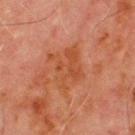biopsy_status: not biopsied; imaged during a skin examination
patient:
  sex: male
  age_approx: 70
site: chest
lesion_size:
  long_diameter_mm_approx: 4.5
image:
  source: total-body photography crop
  field_of_view_mm: 15
lighting: cross-polarized